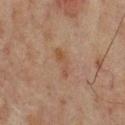Assessment: Part of a total-body skin-imaging series; this lesion was reviewed on a skin check and was not flagged for biopsy. Context: On the back. An algorithmic analysis of the crop reported a footprint of about 3.5 mm², an outline eccentricity of about 0.95 (0 = round, 1 = elongated), and a shape-asymmetry score of about 0.5 (0 = symmetric). It also reported a mean CIELAB color near L≈39 a*≈16 b*≈26, roughly 5 lightness units darker than nearby skin, and a lesion-to-skin contrast of about 5.5 (normalized; higher = more distinct). The patient is a male approximately 65 years of age. The lesion's longest dimension is about 3.5 mm. A roughly 15 mm field-of-view crop from a total-body skin photograph. This is a cross-polarized tile.